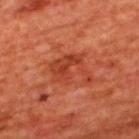workup = no biopsy performed (imaged during a skin exam) | site = the upper back | size = ≈5 mm | acquisition = ~15 mm tile from a whole-body skin photo | subject = male, about 65 years old | illumination = cross-polarized illumination.